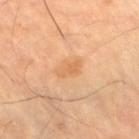workup = total-body-photography surveillance lesion; no biopsy
body site = the right thigh
acquisition = total-body-photography crop, ~15 mm field of view
lesion diameter = ~3 mm (longest diameter)
patient = aged around 65
lighting = cross-polarized